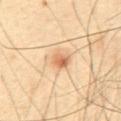workup=catalogued during a skin exam; not biopsied | subject=male, aged around 65 | acquisition=15 mm crop, total-body photography | site=the upper back | illumination=cross-polarized | automated metrics=roughly 12 lightness units darker than nearby skin and a normalized lesion–skin contrast near 7.